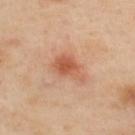The total-body-photography lesion software estimated a lesion color around L≈58 a*≈26 b*≈34 in CIELAB, a lesion–skin lightness drop of about 10, and a lesion-to-skin contrast of about 7 (normalized; higher = more distinct). The software also gave a border-irregularity rating of about 4/10, internal color variation of about 4.5 on a 0–10 scale, and radial color variation of about 1.5. Located on the upper back. Cropped from a whole-body photographic skin survey; the tile spans about 15 mm. This is a cross-polarized tile. A female subject, aged around 40.